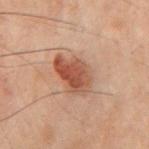Impression:
Imaged during a routine full-body skin examination; the lesion was not biopsied and no histopathology is available.
Background:
Cropped from a total-body skin-imaging series; the visible field is about 15 mm. The subject is a male about 70 years old. The lesion is on the chest.